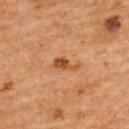<tbp_lesion>
  <biopsy_status>not biopsied; imaged during a skin examination</biopsy_status>
  <image>
    <source>total-body photography crop</source>
    <field_of_view_mm>15</field_of_view_mm>
  </image>
  <lighting>cross-polarized</lighting>
  <lesion_size>
    <long_diameter_mm_approx>3.0</long_diameter_mm_approx>
  </lesion_size>
  <patient>
    <sex>female</sex>
    <age_approx>60</age_approx>
  </patient>
  <site>upper back</site>
</tbp_lesion>